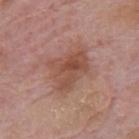Clinical impression: Part of a total-body skin-imaging series; this lesion was reviewed on a skin check and was not flagged for biopsy. Image and clinical context: The lesion-visualizer software estimated a lesion color around L≈50 a*≈22 b*≈27 in CIELAB, roughly 9 lightness units darker than nearby skin, and a lesion-to-skin contrast of about 6.5 (normalized; higher = more distinct). A close-up tile cropped from a whole-body skin photograph, about 15 mm across. The lesion's longest dimension is about 5.5 mm. A male patient aged approximately 75. On the mid back.